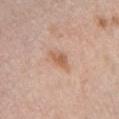| key | value |
|---|---|
| notes | total-body-photography surveillance lesion; no biopsy |
| patient | female, in their mid- to late 60s |
| image | 15 mm crop, total-body photography |
| image-analysis metrics | a lesion area of about 3.5 mm², an eccentricity of roughly 0.8, and a symmetry-axis asymmetry near 0.3; a lesion color around L≈60 a*≈21 b*≈32 in CIELAB, a lesion–skin lightness drop of about 10, and a normalized border contrast of about 7; a within-lesion color-variation index near 2/10 and radial color variation of about 0.5 |
| site | the chest |